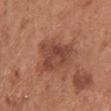Case summary:
- notes — total-body-photography surveillance lesion; no biopsy
- automated metrics — a lesion color around L≈44 a*≈24 b*≈28 in CIELAB, about 10 CIELAB-L* units darker than the surrounding skin, and a normalized lesion–skin contrast near 7.5; border irregularity of about 5 on a 0–10 scale, a color-variation rating of about 4/10, and a peripheral color-asymmetry measure near 1.5; a classifier nevus-likeness of about 25/100 and a detector confidence of about 100 out of 100 that the crop contains a lesion
- illumination — white-light illumination
- subject — female, in their mid- to late 60s
- location — the chest
- lesion diameter — ~5 mm (longest diameter)
- image source — ~15 mm tile from a whole-body skin photo